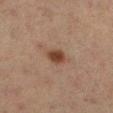<tbp_lesion>
<biopsy_status>not biopsied; imaged during a skin examination</biopsy_status>
<image>
  <source>total-body photography crop</source>
  <field_of_view_mm>15</field_of_view_mm>
</image>
<site>left lower leg</site>
<lighting>cross-polarized</lighting>
<patient>
  <sex>female</sex>
  <age_approx>55</age_approx>
</patient>
<automated_metrics>
  <area_mm2_approx>4.5</area_mm2_approx>
  <eccentricity>0.6</eccentricity>
  <cielab_L>34</cielab_L>
  <cielab_a>17</cielab_a>
  <cielab_b>24</cielab_b>
  <vs_skin_darker_L>9.0</vs_skin_darker_L>
  <vs_skin_contrast_norm>9.5</vs_skin_contrast_norm>
  <lesion_detection_confidence_0_100>100</lesion_detection_confidence_0_100>
</automated_metrics>
<lesion_size>
  <long_diameter_mm_approx>2.5</long_diameter_mm_approx>
</lesion_size>
</tbp_lesion>